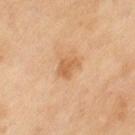<record>
<biopsy_status>not biopsied; imaged during a skin examination</biopsy_status>
<site>left thigh</site>
<patient>
  <sex>female</sex>
  <age_approx>60</age_approx>
</patient>
<lesion_size>
  <long_diameter_mm_approx>3.0</long_diameter_mm_approx>
</lesion_size>
<image>
  <source>total-body photography crop</source>
  <field_of_view_mm>15</field_of_view_mm>
</image>
<lighting>cross-polarized</lighting>
<automated_metrics>
  <cielab_L>56</cielab_L>
  <cielab_a>20</cielab_a>
  <cielab_b>37</cielab_b>
  <vs_skin_darker_L>8.0</vs_skin_darker_L>
  <vs_skin_contrast_norm>6.5</vs_skin_contrast_norm>
  <nevus_likeness_0_100>0</nevus_likeness_0_100>
</automated_metrics>
</record>